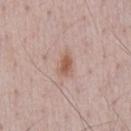Findings:
• follow-up: total-body-photography surveillance lesion; no biopsy
• body site: the chest
• image source: total-body-photography crop, ~15 mm field of view
• lesion diameter: ~2.5 mm (longest diameter)
• automated lesion analysis: radial color variation of about 0.5; an automated nevus-likeness rating near 80 out of 100 and lesion-presence confidence of about 100/100
• lighting: white-light illumination
• patient: male, aged around 50